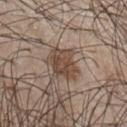  biopsy_status: not biopsied; imaged during a skin examination
  image:
    source: total-body photography crop
    field_of_view_mm: 15
  automated_metrics:
    cielab_L: 46
    cielab_a: 15
    cielab_b: 25
    vs_skin_darker_L: 11.0
    vs_skin_contrast_norm: 8.5
    border_irregularity_0_10: 3.0
    color_variation_0_10: 4.0
    peripheral_color_asymmetry: 1.5
    nevus_likeness_0_100: 70
    lesion_detection_confidence_0_100: 100
  patient:
    sex: male
    age_approx: 60
  lighting: white-light
  site: chest
  lesion_size:
    long_diameter_mm_approx: 4.5